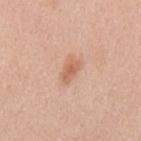Q: Was this lesion biopsied?
A: imaged on a skin check; not biopsied
Q: Where on the body is the lesion?
A: the mid back
Q: What kind of image is this?
A: total-body-photography crop, ~15 mm field of view
Q: What did automated image analysis measure?
A: a lesion color around L≈63 a*≈23 b*≈33 in CIELAB and a lesion-to-skin contrast of about 6.5 (normalized; higher = more distinct)
Q: Patient demographics?
A: female, aged 38 to 42
Q: Illumination type?
A: white-light illumination